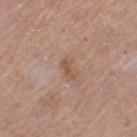Longest diameter approximately 3 mm.
Cropped from a total-body skin-imaging series; the visible field is about 15 mm.
The lesion is on the leg.
This is a white-light tile.
A female subject aged 58 to 62.
Automated tile analysis of the lesion measured a footprint of about 3 mm² and an eccentricity of roughly 0.85. The software also gave a mean CIELAB color near L≈53 a*≈19 b*≈29, a lesion–skin lightness drop of about 7, and a normalized lesion–skin contrast near 6. And it measured a nevus-likeness score of about 0/100.